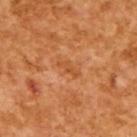Clinical impression: Imaged during a routine full-body skin examination; the lesion was not biopsied and no histopathology is available. Background: A 15 mm close-up extracted from a 3D total-body photography capture. The tile uses cross-polarized illumination. The patient is a male about 65 years old.